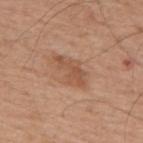follow-up = imaged on a skin check; not biopsied.